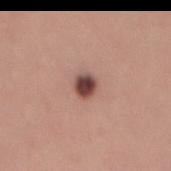site: the mid back | imaging modality: 15 mm crop, total-body photography | patient: female, in their mid- to late 20s | image-analysis metrics: a mean CIELAB color near L≈45 a*≈21 b*≈23, a lesion–skin lightness drop of about 18, and a normalized border contrast of about 12.5; border irregularity of about 2 on a 0–10 scale, a color-variation rating of about 4.5/10, and peripheral color asymmetry of about 1.5 | tile lighting: white-light illumination | lesion diameter: ~2.5 mm (longest diameter).